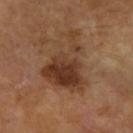Impression:
Captured during whole-body skin photography for melanoma surveillance; the lesion was not biopsied.
Acquisition and patient details:
The tile uses cross-polarized illumination. About 7.5 mm across. Automated image analysis of the tile measured a mean CIELAB color near L≈38 a*≈20 b*≈30 and about 11 CIELAB-L* units darker than the surrounding skin. The software also gave a border-irregularity rating of about 7/10, a color-variation rating of about 6/10, and a peripheral color-asymmetry measure near 2. The software also gave an automated nevus-likeness rating near 30 out of 100. On the right forearm. A 15 mm crop from a total-body photograph taken for skin-cancer surveillance. A female subject approximately 70 years of age.